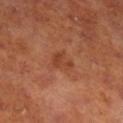Imaged during a routine full-body skin examination; the lesion was not biopsied and no histopathology is available.
A male subject roughly 70 years of age.
The total-body-photography lesion software estimated lesion-presence confidence of about 100/100.
A 15 mm close-up tile from a total-body photography series done for melanoma screening.
The recorded lesion diameter is about 2.5 mm.
Located on the right lower leg.